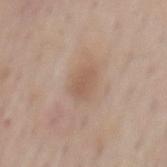Notes:
– anatomic site · the mid back
– patient · male, approximately 75 years of age
– size · ≈3 mm
– image · ~15 mm tile from a whole-body skin photo
– TBP lesion metrics · a footprint of about 5 mm², an eccentricity of roughly 0.7, and a symmetry-axis asymmetry near 0.2; a mean CIELAB color near L≈56 a*≈17 b*≈28 and a lesion–skin lightness drop of about 7; a border-irregularity rating of about 2/10, a within-lesion color-variation index near 1/10, and peripheral color asymmetry of about 0.5